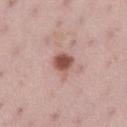Case summary:
* follow-up · imaged on a skin check; not biopsied
* body site · the left lower leg
* image · ~15 mm crop, total-body skin-cancer survey
* subject · female, aged around 35